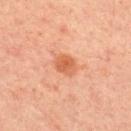No biopsy was performed on this lesion — it was imaged during a full skin examination and was not determined to be concerning.
Longest diameter approximately 3 mm.
From the upper back.
A male subject aged 28 to 32.
Imaged with cross-polarized lighting.
An algorithmic analysis of the crop reported a footprint of about 5.5 mm² and two-axis asymmetry of about 0.15. And it measured an average lesion color of about L≈50 a*≈25 b*≈33 (CIELAB), a lesion–skin lightness drop of about 9, and a normalized lesion–skin contrast near 7. And it measured a nevus-likeness score of about 90/100 and a lesion-detection confidence of about 100/100.
Cropped from a total-body skin-imaging series; the visible field is about 15 mm.